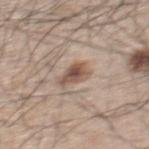| field | value |
|---|---|
| notes | catalogued during a skin exam; not biopsied |
| lighting | white-light illumination |
| patient | male, roughly 65 years of age |
| acquisition | total-body-photography crop, ~15 mm field of view |
| site | the back |